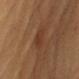| feature | finding |
|---|---|
| biopsy status | total-body-photography surveillance lesion; no biopsy |
| subject | male, aged 83 or older |
| image | ~15 mm tile from a whole-body skin photo |
| site | the left upper arm |